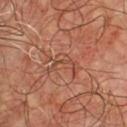This lesion was catalogued during total-body skin photography and was not selected for biopsy. A male patient in their mid-50s. A roughly 15 mm field-of-view crop from a total-body skin photograph. The lesion is located on the chest. The lesion's longest dimension is about 3.5 mm. An algorithmic analysis of the crop reported a footprint of about 4.5 mm², an outline eccentricity of about 0.8 (0 = round, 1 = elongated), and a shape-asymmetry score of about 0.85 (0 = symmetric). And it measured a lesion color around L≈47 a*≈24 b*≈31 in CIELAB, a lesion–skin lightness drop of about 6, and a normalized lesion–skin contrast near 5. And it measured a border-irregularity index near 9.5/10, a within-lesion color-variation index near 1/10, and a peripheral color-asymmetry measure near 0.5. The software also gave a classifier nevus-likeness of about 0/100 and lesion-presence confidence of about 80/100. Captured under cross-polarized illumination.